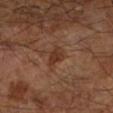Clinical impression: No biopsy was performed on this lesion — it was imaged during a full skin examination and was not determined to be concerning. Context: The lesion's longest dimension is about 3 mm. Cropped from a whole-body photographic skin survey; the tile spans about 15 mm. Automated image analysis of the tile measured a border-irregularity rating of about 2/10, a color-variation rating of about 2/10, and a peripheral color-asymmetry measure near 0.5. A subject in their mid-60s. On the left lower leg.